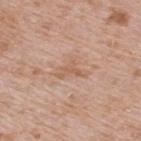The lesion was tiled from a total-body skin photograph and was not biopsied.
Cropped from a total-body skin-imaging series; the visible field is about 15 mm.
The lesion's longest dimension is about 3.5 mm.
The patient is a male aged around 65.
The lesion is on the upper back.